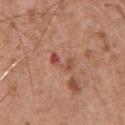Captured during whole-body skin photography for melanoma surveillance; the lesion was not biopsied. This is a white-light tile. Automated tile analysis of the lesion measured a footprint of about 4 mm², an outline eccentricity of about 0.9 (0 = round, 1 = elongated), and a shape-asymmetry score of about 0.45 (0 = symmetric). It also reported an average lesion color of about L≈51 a*≈27 b*≈30 (CIELAB), a lesion–skin lightness drop of about 9, and a lesion-to-skin contrast of about 6.5 (normalized; higher = more distinct). It also reported border irregularity of about 6 on a 0–10 scale and peripheral color asymmetry of about 0.5. The subject is a male about 75 years old. A roughly 15 mm field-of-view crop from a total-body skin photograph. The lesion is located on the front of the torso.